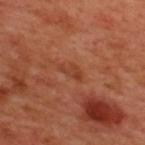No biopsy was performed on this lesion — it was imaged during a full skin examination and was not determined to be concerning.
Located on the back.
The subject is a male in their 50s.
A 15 mm close-up tile from a total-body photography series done for melanoma screening.
The recorded lesion diameter is about 3 mm.